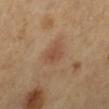Imaged during a routine full-body skin examination; the lesion was not biopsied and no histopathology is available. Captured under cross-polarized illumination. A roughly 15 mm field-of-view crop from a total-body skin photograph. From the left lower leg. A female subject aged 58 to 62. Longest diameter approximately 2.5 mm.